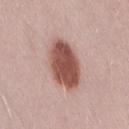The recorded lesion diameter is about 6 mm. From the right thigh. Captured under white-light illumination. The subject is a male approximately 55 years of age. Automated tile analysis of the lesion measured an automated nevus-likeness rating near 100 out of 100 and lesion-presence confidence of about 100/100. A lesion tile, about 15 mm wide, cut from a 3D total-body photograph.